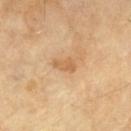follow-up = imaged on a skin check; not biopsied
lesion size = ≈2.5 mm
acquisition = 15 mm crop, total-body photography
patient = male, in their mid- to late 60s